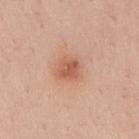Recorded during total-body skin imaging; not selected for excision or biopsy. A male patient, aged 28 to 32. The lesion's longest dimension is about 2.5 mm. A region of skin cropped from a whole-body photographic capture, roughly 15 mm wide. Located on the mid back.